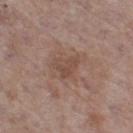This lesion was catalogued during total-body skin photography and was not selected for biopsy. This is a white-light tile. A 15 mm close-up tile from a total-body photography series done for melanoma screening. A female subject aged 83 or over. Located on the right thigh. An algorithmic analysis of the crop reported an area of roughly 5.5 mm², a shape eccentricity near 0.65, and a shape-asymmetry score of about 0.4 (0 = symmetric). It also reported a peripheral color-asymmetry measure near 1. It also reported a nevus-likeness score of about 0/100 and a detector confidence of about 100 out of 100 that the crop contains a lesion.